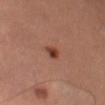size: ≈2 mm; lighting: cross-polarized; imaging modality: total-body-photography crop, ~15 mm field of view; subject: female, in their mid- to late 40s; site: the left thigh.